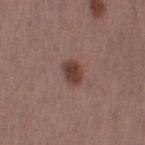Q: Lesion size?
A: ≈2.5 mm
Q: How was this image acquired?
A: ~15 mm tile from a whole-body skin photo
Q: Illumination type?
A: white-light illumination
Q: What are the patient's age and sex?
A: female, aged 48–52
Q: Automated lesion metrics?
A: a lesion area of about 5 mm² and a symmetry-axis asymmetry near 0.2; a border-irregularity index near 2/10, internal color variation of about 2 on a 0–10 scale, and peripheral color asymmetry of about 0.5; a classifier nevus-likeness of about 95/100
Q: Lesion location?
A: the left thigh